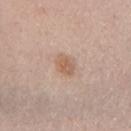Impression:
Part of a total-body skin-imaging series; this lesion was reviewed on a skin check and was not flagged for biopsy.
Image and clinical context:
A female subject roughly 40 years of age. Captured under white-light illumination. The recorded lesion diameter is about 3 mm. On the right upper arm. This image is a 15 mm lesion crop taken from a total-body photograph.